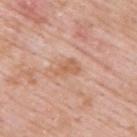Imaged during a routine full-body skin examination; the lesion was not biopsied and no histopathology is available. The total-body-photography lesion software estimated an average lesion color of about L≈61 a*≈22 b*≈33 (CIELAB), a lesion–skin lightness drop of about 8, and a lesion-to-skin contrast of about 6 (normalized; higher = more distinct). The software also gave border irregularity of about 3 on a 0–10 scale and internal color variation of about 1.5 on a 0–10 scale. And it measured an automated nevus-likeness rating near 0 out of 100 and lesion-presence confidence of about 100/100. A male patient aged 53–57. The lesion's longest dimension is about 3 mm. Located on the upper back. The tile uses white-light illumination. A region of skin cropped from a whole-body photographic capture, roughly 15 mm wide.